This is a cross-polarized tile · this image is a 15 mm lesion crop taken from a total-body photograph · the recorded lesion diameter is about 4.5 mm · a female patient, approximately 60 years of age · the lesion is on the chest · Automated tile analysis of the lesion measured a lesion area of about 8 mm², an outline eccentricity of about 0.85 (0 = round, 1 = elongated), and a symmetry-axis asymmetry near 0.3. The analysis additionally found internal color variation of about 4 on a 0–10 scale and peripheral color asymmetry of about 1.5. It also reported a lesion-detection confidence of about 100/100.
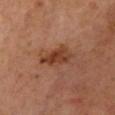Q: What is the histopathologic diagnosis?
A: a melanoma in situ